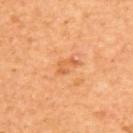Captured under cross-polarized illumination. The recorded lesion diameter is about 3 mm. A female patient roughly 55 years of age. The lesion is on the upper back. A 15 mm close-up tile from a total-body photography series done for melanoma screening.